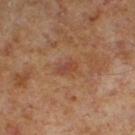Clinical summary: This is a cross-polarized tile. Automated tile analysis of the lesion measured an average lesion color of about L≈41 a*≈22 b*≈28 (CIELAB) and a normalized border contrast of about 6. The software also gave a border-irregularity rating of about 2.5/10, internal color variation of about 2.5 on a 0–10 scale, and a peripheral color-asymmetry measure near 1. The analysis additionally found a nevus-likeness score of about 15/100. A close-up tile cropped from a whole-body skin photograph, about 15 mm across. On the left lower leg. A male subject, roughly 60 years of age. Longest diameter approximately 2.5 mm.